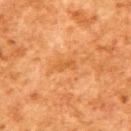<case>
  <lighting>cross-polarized</lighting>
  <patient>
    <sex>male</sex>
    <age_approx>80</age_approx>
  </patient>
  <image>
    <source>total-body photography crop</source>
    <field_of_view_mm>15</field_of_view_mm>
  </image>
  <site>upper back</site>
</case>